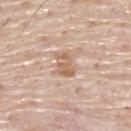biopsy status — imaged on a skin check; not biopsied
size — ≈3 mm
image — ~15 mm crop, total-body skin-cancer survey
illumination — white-light illumination
subject — male, approximately 80 years of age
body site — the upper back
automated metrics — a lesion area of about 5 mm², an outline eccentricity of about 0.7 (0 = round, 1 = elongated), and a shape-asymmetry score of about 0.3 (0 = symmetric); about 10 CIELAB-L* units darker than the surrounding skin and a lesion-to-skin contrast of about 7 (normalized; higher = more distinct)